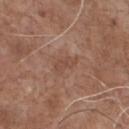image:
  source: total-body photography crop
  field_of_view_mm: 15
automated_metrics:
  eccentricity: 0.85
  cielab_L: 47
  cielab_a: 20
  cielab_b: 27
  vs_skin_darker_L: 6.0
  vs_skin_contrast_norm: 4.5
  border_irregularity_0_10: 4.0
  color_variation_0_10: 1.5
  peripheral_color_asymmetry: 0.5
  nevus_likeness_0_100: 0
site: chest
lesion_size:
  long_diameter_mm_approx: 3.0
patient:
  sex: male
  age_approx: 70